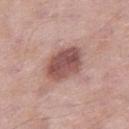The lesion was tiled from a total-body skin photograph and was not biopsied.
A male patient, approximately 55 years of age.
This image is a 15 mm lesion crop taken from a total-body photograph.
The lesion is located on the leg.